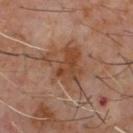Notes:
– biopsy status · no biopsy performed (imaged during a skin exam)
– site · the front of the torso
– diameter · about 6.5 mm
– image-analysis metrics · a lesion area of about 21 mm², an eccentricity of roughly 0.6, and a shape-asymmetry score of about 0.35 (0 = symmetric); a lesion color around L≈42 a*≈19 b*≈28 in CIELAB, roughly 8 lightness units darker than nearby skin, and a normalized lesion–skin contrast near 7; a border-irregularity index near 5/10 and a within-lesion color-variation index near 6/10; a nevus-likeness score of about 0/100
– lighting · cross-polarized
– image · ~15 mm tile from a whole-body skin photo
– subject · male, approximately 60 years of age